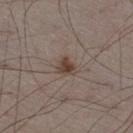Recorded during total-body skin imaging; not selected for excision or biopsy. Imaged with white-light lighting. On the right thigh. Automated tile analysis of the lesion measured a lesion area of about 4 mm² and a symmetry-axis asymmetry near 0.3. The analysis additionally found a border-irregularity index near 2.5/10, a color-variation rating of about 3/10, and radial color variation of about 1. The software also gave a nevus-likeness score of about 90/100 and lesion-presence confidence of about 100/100. A close-up tile cropped from a whole-body skin photograph, about 15 mm across. A male subject, in their mid-70s.